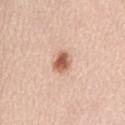| field | value |
|---|---|
| workup | catalogued during a skin exam; not biopsied |
| imaging modality | 15 mm crop, total-body photography |
| TBP lesion metrics | a lesion area of about 5 mm² and two-axis asymmetry of about 0.25; an average lesion color of about L≈61 a*≈23 b*≈32 (CIELAB) and a normalized lesion–skin contrast near 9.5; a border-irregularity rating of about 2/10, a within-lesion color-variation index near 4.5/10, and radial color variation of about 1.5; a nevus-likeness score of about 100/100 |
| location | the abdomen |
| subject | female, in their mid-60s |
| lesion diameter | ≈3 mm |